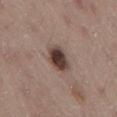This lesion was catalogued during total-body skin photography and was not selected for biopsy.
From the lower back.
Automated tile analysis of the lesion measured a footprint of about 7.5 mm², an eccentricity of roughly 0.65, and two-axis asymmetry of about 0.2. The software also gave internal color variation of about 5.5 on a 0–10 scale and radial color variation of about 1.5. The software also gave a classifier nevus-likeness of about 95/100 and lesion-presence confidence of about 100/100.
Approximately 3.5 mm at its widest.
A male patient aged around 55.
The tile uses white-light illumination.
A close-up tile cropped from a whole-body skin photograph, about 15 mm across.